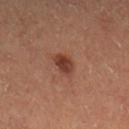Clinical impression: Part of a total-body skin-imaging series; this lesion was reviewed on a skin check and was not flagged for biopsy. Context: Cropped from a total-body skin-imaging series; the visible field is about 15 mm. The patient is a male aged around 60. From the right lower leg. Measured at roughly 2.5 mm in maximum diameter.